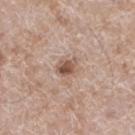Q: Is there a histopathology result?
A: no biopsy performed (imaged during a skin exam)
Q: Patient demographics?
A: male, in their 60s
Q: Lesion location?
A: the left lower leg
Q: How was this image acquired?
A: ~15 mm crop, total-body skin-cancer survey